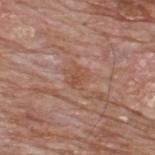{"biopsy_status": "not biopsied; imaged during a skin examination", "patient": {"sex": "male", "age_approx": 60}, "lesion_size": {"long_diameter_mm_approx": 2.5}, "image": {"source": "total-body photography crop", "field_of_view_mm": 15}, "lighting": "white-light", "automated_metrics": {"area_mm2_approx": 4.5, "shape_asymmetry": 0.3, "cielab_L": 51, "cielab_a": 22, "cielab_b": 29, "vs_skin_darker_L": 6.0, "lesion_detection_confidence_0_100": 90}, "site": "upper back"}